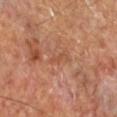Imaged with cross-polarized lighting.
Longest diameter approximately 2.5 mm.
A male patient in their mid-60s.
Located on the right lower leg.
A close-up tile cropped from a whole-body skin photograph, about 15 mm across.
The lesion-visualizer software estimated an automated nevus-likeness rating near 0 out of 100 and a detector confidence of about 100 out of 100 that the crop contains a lesion.